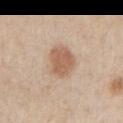Automated tile analysis of the lesion measured about 11 CIELAB-L* units darker than the surrounding skin and a normalized lesion–skin contrast near 7.5.
The recorded lesion diameter is about 4 mm.
The lesion is located on the left upper arm.
A male subject, aged approximately 60.
Cropped from a total-body skin-imaging series; the visible field is about 15 mm.